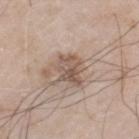notes=total-body-photography surveillance lesion; no biopsy
tile lighting=white-light
size=about 3.5 mm
subject=male, in their 60s
body site=the left thigh
image source=~15 mm crop, total-body skin-cancer survey